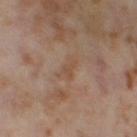A female subject, in their mid-50s. The lesion is located on the right thigh. A close-up tile cropped from a whole-body skin photograph, about 15 mm across. About 3 mm across. The lesion-visualizer software estimated a border-irregularity index near 4.5/10 and a peripheral color-asymmetry measure near 0.